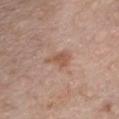Q: Is there a histopathology result?
A: no biopsy performed (imaged during a skin exam)
Q: Where on the body is the lesion?
A: the chest
Q: Automated lesion metrics?
A: a mean CIELAB color near L≈54 a*≈20 b*≈30 and a lesion-to-skin contrast of about 7 (normalized; higher = more distinct); a classifier nevus-likeness of about 5/100
Q: Lesion size?
A: about 3.5 mm
Q: What are the patient's age and sex?
A: male, aged around 55
Q: What kind of image is this?
A: total-body-photography crop, ~15 mm field of view